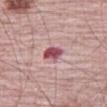Case summary:
* biopsy status: total-body-photography surveillance lesion; no biopsy
* location: the right thigh
* tile lighting: white-light
* subject: male, aged around 70
* diameter: ≈2.5 mm
* image: ~15 mm crop, total-body skin-cancer survey